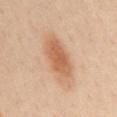biopsy status=total-body-photography surveillance lesion; no biopsy | illumination=cross-polarized illumination | lesion size=about 6.5 mm | automated metrics=a color-variation rating of about 4/10 and peripheral color asymmetry of about 1 | site=the mid back | imaging modality=~15 mm crop, total-body skin-cancer survey | subject=female, aged around 45.